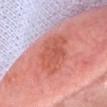  biopsy_status: not biopsied; imaged during a skin examination
  lighting: white-light
  automated_metrics:
    area_mm2_approx: 18.0
    eccentricity: 0.7
    cielab_L: 58
    cielab_a: 32
    cielab_b: 31
    vs_skin_darker_L: 9.0
    vs_skin_contrast_norm: 8.0
  image:
    source: total-body photography crop
    field_of_view_mm: 15
  lesion_size:
    long_diameter_mm_approx: 6.0
  site: head or neck
  patient:
    sex: female
    age_approx: 65